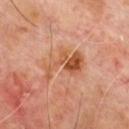workup: total-body-photography surveillance lesion; no biopsy
illumination: cross-polarized illumination
patient: male, in their mid- to late 60s
site: the chest
acquisition: 15 mm crop, total-body photography
lesion size: ≈5.5 mm
TBP lesion metrics: a mean CIELAB color near L≈56 a*≈26 b*≈37 and a normalized lesion–skin contrast near 7.5; a border-irregularity index near 9/10 and peripheral color asymmetry of about 3; a classifier nevus-likeness of about 10/100 and a lesion-detection confidence of about 100/100